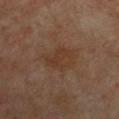Q: Is there a histopathology result?
A: catalogued during a skin exam; not biopsied
Q: What is the anatomic site?
A: the chest
Q: Patient demographics?
A: female, aged approximately 60
Q: What kind of image is this?
A: total-body-photography crop, ~15 mm field of view
Q: Lesion size?
A: ≈5 mm
Q: What lighting was used for the tile?
A: cross-polarized